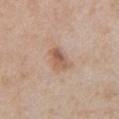Impression:
The lesion was tiled from a total-body skin photograph and was not biopsied.
Context:
From the abdomen. A male subject, in their mid- to late 60s. Automated tile analysis of the lesion measured an eccentricity of roughly 0.8 and two-axis asymmetry of about 0.25. The analysis additionally found an automated nevus-likeness rating near 30 out of 100 and a detector confidence of about 100 out of 100 that the crop contains a lesion. Imaged with white-light lighting. This image is a 15 mm lesion crop taken from a total-body photograph. Measured at roughly 3 mm in maximum diameter.